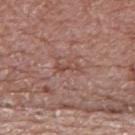Impression:
The lesion was tiled from a total-body skin photograph and was not biopsied.
Clinical summary:
This image is a 15 mm lesion crop taken from a total-body photograph. On the back. A male subject, aged approximately 70. Captured under white-light illumination. The total-body-photography lesion software estimated a lesion area of about 2.5 mm², a shape eccentricity near 0.95, and two-axis asymmetry of about 0.35. Measured at roughly 2.5 mm in maximum diameter.